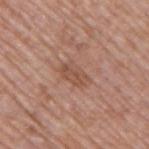biopsy status: imaged on a skin check; not biopsied
anatomic site: the right upper arm
imaging modality: total-body-photography crop, ~15 mm field of view
patient: male, aged around 70